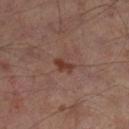Q: Was this lesion biopsied?
A: imaged on a skin check; not biopsied
Q: Automated lesion metrics?
A: an average lesion color of about L≈36 a*≈20 b*≈24 (CIELAB), about 8 CIELAB-L* units darker than the surrounding skin, and a normalized border contrast of about 7.5; a border-irregularity rating of about 2.5/10, a within-lesion color-variation index near 2/10, and a peripheral color-asymmetry measure near 1
Q: Lesion size?
A: ≈2.5 mm
Q: What are the patient's age and sex?
A: male, aged 63 to 67
Q: How was this image acquired?
A: total-body-photography crop, ~15 mm field of view
Q: What is the anatomic site?
A: the left lower leg
Q: What lighting was used for the tile?
A: cross-polarized